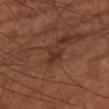Imaged during a routine full-body skin examination; the lesion was not biopsied and no histopathology is available. From the right lower leg. A 15 mm close-up tile from a total-body photography series done for melanoma screening. A patient in their mid- to late 60s.